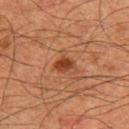biopsy status: no biopsy performed (imaged during a skin exam)
acquisition: ~15 mm tile from a whole-body skin photo
site: the upper back
subject: male, aged approximately 65
TBP lesion metrics: a border-irregularity index near 2.5/10, a within-lesion color-variation index near 2/10, and peripheral color asymmetry of about 0.5; a classifier nevus-likeness of about 95/100 and lesion-presence confidence of about 100/100
lesion size: ≈2.5 mm
lighting: cross-polarized